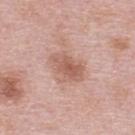* workup · catalogued during a skin exam; not biopsied
* tile lighting · white-light
* subject · female, about 65 years old
* body site · the upper back
* image · ~15 mm tile from a whole-body skin photo